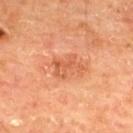Impression:
Recorded during total-body skin imaging; not selected for excision or biopsy.
Background:
Located on the upper back. An algorithmic analysis of the crop reported an area of roughly 6 mm², a shape eccentricity near 0.8, and a symmetry-axis asymmetry near 0.4. The analysis additionally found a lesion–skin lightness drop of about 9 and a lesion-to-skin contrast of about 6 (normalized; higher = more distinct). The analysis additionally found an automated nevus-likeness rating near 5 out of 100 and a lesion-detection confidence of about 100/100. This is a cross-polarized tile. A male patient, about 65 years old. A 15 mm close-up extracted from a 3D total-body photography capture.